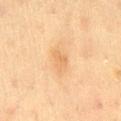No biopsy was performed on this lesion — it was imaged during a full skin examination and was not determined to be concerning. Approximately 3.5 mm at its widest. A male subject about 60 years old. The lesion is located on the lower back. Cropped from a total-body skin-imaging series; the visible field is about 15 mm. An algorithmic analysis of the crop reported a lesion color around L≈59 a*≈17 b*≈36 in CIELAB. The analysis additionally found border irregularity of about 2.5 on a 0–10 scale, a color-variation rating of about 2/10, and a peripheral color-asymmetry measure near 0.5. The software also gave a classifier nevus-likeness of about 0/100 and lesion-presence confidence of about 100/100. This is a cross-polarized tile.